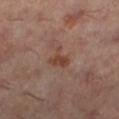• follow-up — total-body-photography surveillance lesion; no biopsy
• site — the left lower leg
• image — 15 mm crop, total-body photography
• image-analysis metrics — internal color variation of about 3 on a 0–10 scale and a peripheral color-asymmetry measure near 1; a classifier nevus-likeness of about 10/100 and lesion-presence confidence of about 100/100
• subject — female, aged around 60